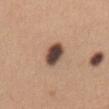follow-up: total-body-photography surveillance lesion; no biopsy
subject: female, in their mid-40s
site: the abdomen
acquisition: ~15 mm tile from a whole-body skin photo
tile lighting: white-light